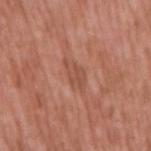Clinical impression: Captured during whole-body skin photography for melanoma surveillance; the lesion was not biopsied. Image and clinical context: The lesion is located on the right upper arm. The subject is a male approximately 55 years of age. The total-body-photography lesion software estimated a footprint of about 5.5 mm², an eccentricity of roughly 0.85, and two-axis asymmetry of about 0.3. The software also gave a mean CIELAB color near L≈51 a*≈25 b*≈30, about 6 CIELAB-L* units darker than the surrounding skin, and a normalized lesion–skin contrast near 4.5. Cropped from a total-body skin-imaging series; the visible field is about 15 mm. About 4 mm across.